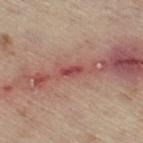| key | value |
|---|---|
| imaging modality | 15 mm crop, total-body photography |
| site | the right thigh |
| TBP lesion metrics | a lesion area of about 3 mm² and two-axis asymmetry of about 0.3 |
| lesion size | about 2.5 mm |
| lighting | white-light |
| subject | male, approximately 70 years of age |